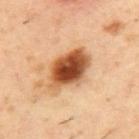{
  "biopsy_status": "not biopsied; imaged during a skin examination"
}